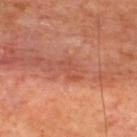biopsy status: imaged on a skin check; not biopsied | image-analysis metrics: a footprint of about 3.5 mm², a shape eccentricity near 0.85, and a symmetry-axis asymmetry near 0.55; a color-variation rating of about 0/10 and radial color variation of about 0; a nevus-likeness score of about 0/100 | imaging modality: ~15 mm tile from a whole-body skin photo | location: the back | patient: male, aged 63 to 67 | size: ~3 mm (longest diameter) | tile lighting: cross-polarized.